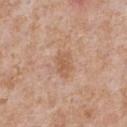This lesion was catalogued during total-body skin photography and was not selected for biopsy.
From the chest.
A roughly 15 mm field-of-view crop from a total-body skin photograph.
The lesion's longest dimension is about 3.5 mm.
This is a white-light tile.
The subject is a male aged 63 to 67.
The total-body-photography lesion software estimated a lesion color around L≈58 a*≈20 b*≈32 in CIELAB, about 8 CIELAB-L* units darker than the surrounding skin, and a lesion-to-skin contrast of about 5.5 (normalized; higher = more distinct). And it measured internal color variation of about 2 on a 0–10 scale and radial color variation of about 0.5.